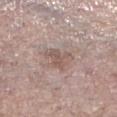Findings:
* notes · no biopsy performed (imaged during a skin exam)
* acquisition · ~15 mm crop, total-body skin-cancer survey
* patient · female, approximately 85 years of age or older
* location · the right lower leg